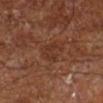Assessment:
Part of a total-body skin-imaging series; this lesion was reviewed on a skin check and was not flagged for biopsy.
Background:
The lesion is on the right lower leg. A close-up tile cropped from a whole-body skin photograph, about 15 mm across. The lesion's longest dimension is about 3 mm. A male patient aged 63–67. Imaged with cross-polarized lighting.